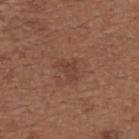notes — no biopsy performed (imaged during a skin exam)
lighting — white-light
automated lesion analysis — an area of roughly 3.5 mm², a shape eccentricity near 0.7, and a shape-asymmetry score of about 0.55 (0 = symmetric); a lesion color around L≈41 a*≈22 b*≈27 in CIELAB, roughly 6 lightness units darker than nearby skin, and a normalized lesion–skin contrast near 5; a within-lesion color-variation index near 1.5/10 and a peripheral color-asymmetry measure near 0.5; an automated nevus-likeness rating near 0 out of 100 and a lesion-detection confidence of about 100/100
lesion size — ~3 mm (longest diameter)
image source — ~15 mm crop, total-body skin-cancer survey
subject — female, aged around 65
site — the back